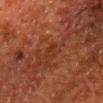Impression:
Recorded during total-body skin imaging; not selected for excision or biopsy.
Image and clinical context:
This image is a 15 mm lesion crop taken from a total-body photograph. Imaged with cross-polarized lighting. Measured at roughly 2.5 mm in maximum diameter. On the head or neck. An algorithmic analysis of the crop reported border irregularity of about 3.5 on a 0–10 scale and internal color variation of about 0.5 on a 0–10 scale. A male patient roughly 60 years of age.